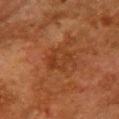Q: Was this lesion biopsied?
A: catalogued during a skin exam; not biopsied
Q: What kind of image is this?
A: ~15 mm crop, total-body skin-cancer survey
Q: What did automated image analysis measure?
A: a footprint of about 7 mm² and a symmetry-axis asymmetry near 0.25; a classifier nevus-likeness of about 0/100 and lesion-presence confidence of about 100/100
Q: How was the tile lit?
A: cross-polarized
Q: Patient demographics?
A: female, aged approximately 50
Q: What is the anatomic site?
A: the chest